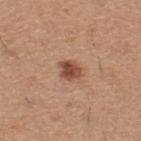Notes:
• notes: catalogued during a skin exam; not biopsied
• size: ≈3 mm
• image source: ~15 mm crop, total-body skin-cancer survey
• automated metrics: a lesion-detection confidence of about 100/100
• subject: male, in their 60s
• tile lighting: white-light illumination
• body site: the upper back